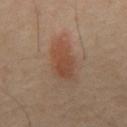Clinical impression:
Part of a total-body skin-imaging series; this lesion was reviewed on a skin check and was not flagged for biopsy.
Image and clinical context:
Captured under cross-polarized illumination. A male subject roughly 45 years of age. A 15 mm close-up tile from a total-body photography series done for melanoma screening. Longest diameter approximately 6 mm. Located on the mid back.